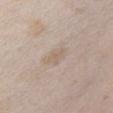Case summary:
- notes: catalogued during a skin exam; not biopsied
- site: the chest
- imaging modality: 15 mm crop, total-body photography
- patient: female, aged around 25
- lighting: white-light illumination